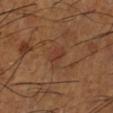Findings:
* notes: catalogued during a skin exam; not biopsied
* subject: male, aged approximately 65
* anatomic site: the leg
* acquisition: ~15 mm tile from a whole-body skin photo
* size: ~3 mm (longest diameter)
* image-analysis metrics: a lesion color around L≈37 a*≈21 b*≈30 in CIELAB, roughly 5 lightness units darker than nearby skin, and a normalized border contrast of about 4.5; a within-lesion color-variation index near 2/10 and peripheral color asymmetry of about 1